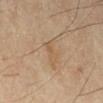biopsy status = catalogued during a skin exam; not biopsied | location = the right lower leg | subject = female, in their 70s | size = about 3 mm | image source = ~15 mm tile from a whole-body skin photo.